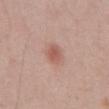Findings:
• follow-up — no biopsy performed (imaged during a skin exam)
• lighting — white-light illumination
• patient — male, aged 53 to 57
• lesion diameter — ~2.5 mm (longest diameter)
• imaging modality — ~15 mm crop, total-body skin-cancer survey
• site — the abdomen
• TBP lesion metrics — an area of roughly 5 mm², a shape eccentricity near 0.6, and a symmetry-axis asymmetry near 0.15; an average lesion color of about L≈57 a*≈23 b*≈27 (CIELAB), about 9 CIELAB-L* units darker than the surrounding skin, and a normalized lesion–skin contrast near 6.5; a border-irregularity index near 1.5/10, internal color variation of about 2.5 on a 0–10 scale, and radial color variation of about 1; an automated nevus-likeness rating near 85 out of 100 and lesion-presence confidence of about 100/100